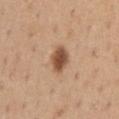  biopsy_status: not biopsied; imaged during a skin examination